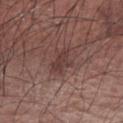Notes:
• biopsy status · no biopsy performed (imaged during a skin exam)
• diameter · ~3 mm (longest diameter)
• acquisition · total-body-photography crop, ~15 mm field of view
• subject · male, aged 73–77
• illumination · white-light
• anatomic site · the arm
• image-analysis metrics · lesion-presence confidence of about 100/100